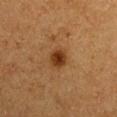| feature | finding |
|---|---|
| workup | catalogued during a skin exam; not biopsied |
| location | the right upper arm |
| illumination | cross-polarized illumination |
| lesion diameter | about 2.5 mm |
| image | total-body-photography crop, ~15 mm field of view |
| image-analysis metrics | an area of roughly 4.5 mm² and two-axis asymmetry of about 0.2; a nevus-likeness score of about 100/100 |
| subject | male, approximately 75 years of age |